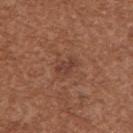<tbp_lesion>
  <patient>
    <sex>female</sex>
    <age_approx>40</age_approx>
  </patient>
  <site>upper back</site>
  <image>
    <source>total-body photography crop</source>
    <field_of_view_mm>15</field_of_view_mm>
  </image>
</tbp_lesion>